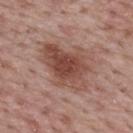Findings:
* notes — catalogued during a skin exam; not biopsied
* diameter — about 6.5 mm
* anatomic site — the upper back
* subject — male, aged 63–67
* automated lesion analysis — a lesion area of about 19 mm², a shape eccentricity near 0.75, and two-axis asymmetry of about 0.35
* tile lighting — white-light
* image — ~15 mm crop, total-body skin-cancer survey